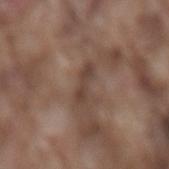biopsy status = imaged on a skin check; not biopsied
lighting = white-light illumination
anatomic site = the lower back
image = 15 mm crop, total-body photography
image-analysis metrics = a shape eccentricity near 0.95 and two-axis asymmetry of about 0.3; a border-irregularity index near 4.5/10, a within-lesion color-variation index near 0/10, and radial color variation of about 0
patient = male, aged around 75
size = ~3.5 mm (longest diameter)